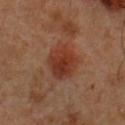A male patient, approximately 50 years of age. On the chest. A region of skin cropped from a whole-body photographic capture, roughly 15 mm wide.